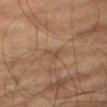Impression:
This lesion was catalogued during total-body skin photography and was not selected for biopsy.
Context:
A male patient aged approximately 80. A close-up tile cropped from a whole-body skin photograph, about 15 mm across. Measured at roughly 2.5 mm in maximum diameter. The lesion is located on the right thigh. Captured under cross-polarized illumination.